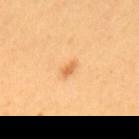follow-up: catalogued during a skin exam; not biopsied
subject: female, roughly 35 years of age
lighting: cross-polarized
acquisition: ~15 mm crop, total-body skin-cancer survey
lesion size: ≈2 mm
site: the upper back
TBP lesion metrics: a footprint of about 2 mm², a shape eccentricity near 0.85, and two-axis asymmetry of about 0.35